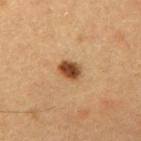Recorded during total-body skin imaging; not selected for excision or biopsy. Captured under cross-polarized illumination. A roughly 15 mm field-of-view crop from a total-body skin photograph. The subject is a male aged 58 to 62. Automated tile analysis of the lesion measured a border-irregularity index near 1.5/10 and peripheral color asymmetry of about 1.5. The analysis additionally found an automated nevus-likeness rating near 100 out of 100 and a detector confidence of about 100 out of 100 that the crop contains a lesion. Approximately 3 mm at its widest.